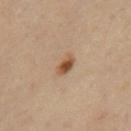{"biopsy_status": "not biopsied; imaged during a skin examination", "patient": {"sex": "female", "age_approx": 40}, "lesion_size": {"long_diameter_mm_approx": 3.0}, "lighting": "cross-polarized", "image": {"source": "total-body photography crop", "field_of_view_mm": 15}, "site": "right thigh"}